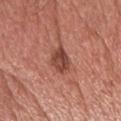biopsy status = total-body-photography surveillance lesion; no biopsy
acquisition = total-body-photography crop, ~15 mm field of view
patient = male, aged around 55
tile lighting = white-light illumination
site = the head or neck